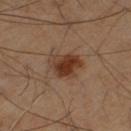Captured during whole-body skin photography for melanoma surveillance; the lesion was not biopsied. A roughly 15 mm field-of-view crop from a total-body skin photograph. Automated image analysis of the tile measured an average lesion color of about L≈32 a*≈18 b*≈26 (CIELAB), a lesion–skin lightness drop of about 10, and a lesion-to-skin contrast of about 10 (normalized; higher = more distinct). And it measured border irregularity of about 2.5 on a 0–10 scale, internal color variation of about 6 on a 0–10 scale, and peripheral color asymmetry of about 2. It also reported a nevus-likeness score of about 95/100 and lesion-presence confidence of about 100/100. The tile uses cross-polarized illumination. The lesion is located on the right thigh. The lesion's longest dimension is about 4 mm. A male subject, aged 58 to 62.